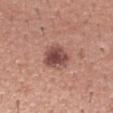Part of a total-body skin-imaging series; this lesion was reviewed on a skin check and was not flagged for biopsy.